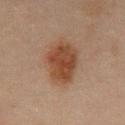Imaged during a routine full-body skin examination; the lesion was not biopsied and no histopathology is available. The tile uses cross-polarized illumination. This image is a 15 mm lesion crop taken from a total-body photograph. The patient is a male aged 43–47. From the front of the torso. The lesion's longest dimension is about 5.5 mm.